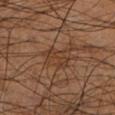Impression:
This lesion was catalogued during total-body skin photography and was not selected for biopsy.
Image and clinical context:
The patient is a male about 60 years old. The lesion is on the left lower leg. A 15 mm crop from a total-body photograph taken for skin-cancer surveillance.